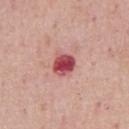Impression: Part of a total-body skin-imaging series; this lesion was reviewed on a skin check and was not flagged for biopsy. Background: This is a white-light tile. About 3 mm across. A male patient, aged 73–77. This image is a 15 mm lesion crop taken from a total-body photograph. The lesion is on the chest.